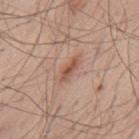<lesion>
<biopsy_status>not biopsied; imaged during a skin examination</biopsy_status>
<patient>
  <sex>male</sex>
  <age_approx>55</age_approx>
</patient>
<site>back</site>
<lighting>white-light</lighting>
<image>
  <source>total-body photography crop</source>
  <field_of_view_mm>15</field_of_view_mm>
</image>
</lesion>